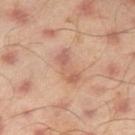workup = total-body-photography surveillance lesion; no biopsy | imaging modality = 15 mm crop, total-body photography | body site = the leg | patient = male, aged around 45.